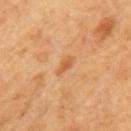* biopsy status · no biopsy performed (imaged during a skin exam)
* tile lighting · cross-polarized illumination
* patient · male, aged approximately 65
* image · 15 mm crop, total-body photography
* anatomic site · the mid back
* size · ≈2.5 mm